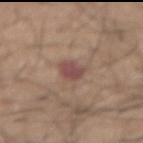Part of a total-body skin-imaging series; this lesion was reviewed on a skin check and was not flagged for biopsy. The patient is a male aged 73–77. From the abdomen. Cropped from a whole-body photographic skin survey; the tile spans about 15 mm.